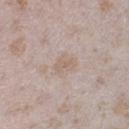• biopsy status: total-body-photography surveillance lesion; no biopsy
• subject: female, aged approximately 25
• lesion size: ≈3 mm
• anatomic site: the right lower leg
• imaging modality: 15 mm crop, total-body photography
• illumination: white-light